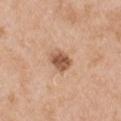Recorded during total-body skin imaging; not selected for excision or biopsy.
Cropped from a total-body skin-imaging series; the visible field is about 15 mm.
Automated image analysis of the tile measured a lesion color around L≈56 a*≈21 b*≈33 in CIELAB, roughly 14 lightness units darker than nearby skin, and a normalized lesion–skin contrast near 9. The analysis additionally found internal color variation of about 4 on a 0–10 scale.
Imaged with white-light lighting.
A male patient aged approximately 50.
The lesion is located on the arm.
Longest diameter approximately 3 mm.